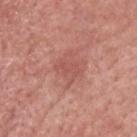Q: Is there a histopathology result?
A: total-body-photography surveillance lesion; no biopsy
Q: Lesion size?
A: ≈3 mm
Q: What kind of image is this?
A: 15 mm crop, total-body photography
Q: Patient demographics?
A: male, approximately 50 years of age
Q: Lesion location?
A: the head or neck
Q: Illumination type?
A: white-light illumination